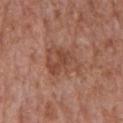Captured during whole-body skin photography for melanoma surveillance; the lesion was not biopsied. Captured under white-light illumination. The patient is a male aged 63 to 67. Cropped from a total-body skin-imaging series; the visible field is about 15 mm. The lesion is located on the chest. The recorded lesion diameter is about 4.5 mm.